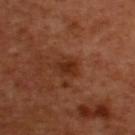Q: Is there a histopathology result?
A: total-body-photography surveillance lesion; no biopsy
Q: What is the anatomic site?
A: the upper back
Q: Illumination type?
A: cross-polarized
Q: How was this image acquired?
A: 15 mm crop, total-body photography
Q: Patient demographics?
A: male, aged 48–52